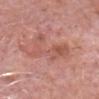Assessment:
Part of a total-body skin-imaging series; this lesion was reviewed on a skin check and was not flagged for biopsy.
Acquisition and patient details:
A roughly 15 mm field-of-view crop from a total-body skin photograph. Measured at roughly 6 mm in maximum diameter. A male subject approximately 80 years of age. The lesion is located on the head or neck. Imaged with white-light lighting.